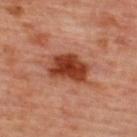{"biopsy_status": "not biopsied; imaged during a skin examination", "image": {"source": "total-body photography crop", "field_of_view_mm": 15}, "lesion_size": {"long_diameter_mm_approx": 5.0}, "lighting": "cross-polarized", "site": "upper back", "patient": {"sex": "female", "age_approx": 45}}